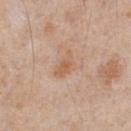biopsy status — total-body-photography surveillance lesion; no biopsy | diameter — ~3.5 mm (longest diameter) | automated lesion analysis — a lesion area of about 5 mm² and two-axis asymmetry of about 0.4; a border-irregularity index near 4/10 and peripheral color asymmetry of about 0.5; a nevus-likeness score of about 5/100 | subject — male, approximately 65 years of age | tile lighting — white-light | body site — the chest | acquisition — 15 mm crop, total-body photography.